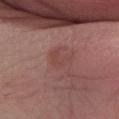Assessment:
The lesion was photographed on a routine skin check and not biopsied; there is no pathology result.
Image and clinical context:
Located on the leg. A female subject about 50 years old. A roughly 15 mm field-of-view crop from a total-body skin photograph. The lesion's longest dimension is about 2.5 mm.